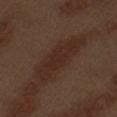Notes:
* follow-up · catalogued during a skin exam; not biopsied
* location · the left upper arm
* image source · 15 mm crop, total-body photography
* TBP lesion metrics · a shape eccentricity near 0.95 and two-axis asymmetry of about 0.3; an average lesion color of about L≈26 a*≈18 b*≈22 (CIELAB); a border-irregularity index near 5.5/10 and a peripheral color-asymmetry measure near 0.5; a nevus-likeness score of about 0/100 and lesion-presence confidence of about 55/100
* illumination · white-light illumination
* patient · male, aged 68 to 72
* lesion size · ~8 mm (longest diameter)